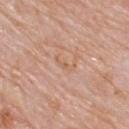| key | value |
|---|---|
| notes | total-body-photography surveillance lesion; no biopsy |
| lesion diameter | ≈3 mm |
| acquisition | 15 mm crop, total-body photography |
| subject | male, aged 73–77 |
| site | the upper back |